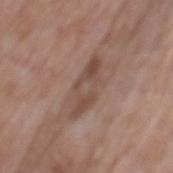Captured during whole-body skin photography for melanoma surveillance; the lesion was not biopsied. The lesion is on the back. A roughly 15 mm field-of-view crop from a total-body skin photograph. Longest diameter approximately 6 mm. The tile uses white-light illumination. A male subject, approximately 65 years of age.